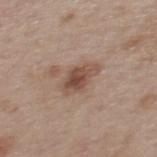biopsy status: imaged on a skin check; not biopsied | image: 15 mm crop, total-body photography | location: the back | TBP lesion metrics: an area of roughly 7.5 mm² and an outline eccentricity of about 0.8 (0 = round, 1 = elongated) | subject: female, about 40 years old | tile lighting: white-light.